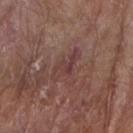The lesion was photographed on a routine skin check and not biopsied; there is no pathology result. Measured at roughly 4 mm in maximum diameter. Imaged with white-light lighting. A male subject, aged approximately 85 or older. The lesion is located on the right forearm. A roughly 15 mm field-of-view crop from a total-body skin photograph.